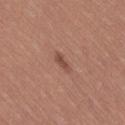Notes:
* biopsy status · imaged on a skin check; not biopsied
* site · the right thigh
* illumination · white-light illumination
* automated metrics · internal color variation of about 0.5 on a 0–10 scale and radial color variation of about 0; a nevus-likeness score of about 0/100 and a lesion-detection confidence of about 100/100
* subject · female, in their 40s
* image source · total-body-photography crop, ~15 mm field of view
* lesion diameter · about 3 mm